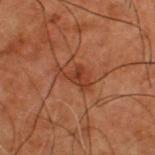{
  "site": "upper back",
  "patient": {
    "sex": "male",
    "age_approx": 50
  },
  "lesion_size": {
    "long_diameter_mm_approx": 4.0
  },
  "image": {
    "source": "total-body photography crop",
    "field_of_view_mm": 15
  },
  "lighting": "cross-polarized"
}